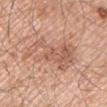Notes:
* biopsy status: total-body-photography surveillance lesion; no biopsy
* site: the arm
* automated metrics: a lesion area of about 19 mm², an outline eccentricity of about 0.85 (0 = round, 1 = elongated), and two-axis asymmetry of about 0.45; a nevus-likeness score of about 0/100
* illumination: white-light
* patient: male, roughly 55 years of age
* acquisition: total-body-photography crop, ~15 mm field of view
* diameter: ≈7.5 mm